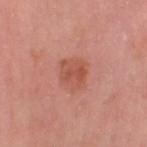notes: no biopsy performed (imaged during a skin exam)
patient: female, aged 53 to 57
size: ~3.5 mm (longest diameter)
body site: the leg
image source: total-body-photography crop, ~15 mm field of view